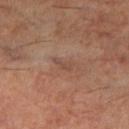This is a cross-polarized tile.
The subject is a male about 60 years old.
A close-up tile cropped from a whole-body skin photograph, about 15 mm across.
The lesion's longest dimension is about 2.5 mm.
On the left lower leg.